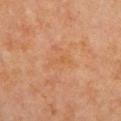follow-up: catalogued during a skin exam; not biopsied
image-analysis metrics: an area of roughly 2.5 mm²; an average lesion color of about L≈47 a*≈20 b*≈33 (CIELAB), a lesion–skin lightness drop of about 4, and a lesion-to-skin contrast of about 4.5 (normalized; higher = more distinct)
imaging modality: 15 mm crop, total-body photography
lighting: cross-polarized
lesion size: about 3 mm
subject: male, aged 68 to 72
body site: the upper back